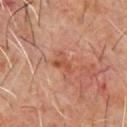Q: Was a biopsy performed?
A: no biopsy performed (imaged during a skin exam)